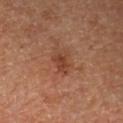follow-up=total-body-photography surveillance lesion; no biopsy | patient=male, aged approximately 65 | automated lesion analysis=a lesion area of about 4 mm² and two-axis asymmetry of about 0.35; a mean CIELAB color near L≈42 a*≈25 b*≈32, about 8 CIELAB-L* units darker than the surrounding skin, and a normalized border contrast of about 7; border irregularity of about 3.5 on a 0–10 scale and a color-variation rating of about 2/10; a nevus-likeness score of about 5/100 and a detector confidence of about 100 out of 100 that the crop contains a lesion | lighting=cross-polarized illumination | anatomic site=the left upper arm | image source=total-body-photography crop, ~15 mm field of view.